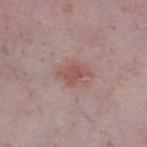<lesion>
<image>
  <source>total-body photography crop</source>
  <field_of_view_mm>15</field_of_view_mm>
</image>
<site>left lower leg</site>
<lighting>white-light</lighting>
<automated_metrics>
  <eccentricity>0.75</eccentricity>
  <shape_asymmetry>0.3</shape_asymmetry>
  <vs_skin_darker_L>8.0</vs_skin_darker_L>
  <vs_skin_contrast_norm>6.5</vs_skin_contrast_norm>
  <border_irregularity_0_10>3.5</border_irregularity_0_10>
  <color_variation_0_10>2.0</color_variation_0_10>
  <peripheral_color_asymmetry>0.5</peripheral_color_asymmetry>
  <nevus_likeness_0_100>65</nevus_likeness_0_100>
</automated_metrics>
<patient>
  <sex>female</sex>
  <age_approx>40</age_approx>
</patient>
<lesion_size>
  <long_diameter_mm_approx>3.5</long_diameter_mm_approx>
</lesion_size>
</lesion>